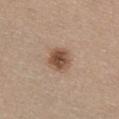Clinical impression:
No biopsy was performed on this lesion — it was imaged during a full skin examination and was not determined to be concerning.
Clinical summary:
A female patient aged around 65. Approximately 3.5 mm at its widest. A roughly 15 mm field-of-view crop from a total-body skin photograph. The lesion is located on the chest. Captured under white-light illumination. Automated tile analysis of the lesion measured an area of roughly 7.5 mm², an eccentricity of roughly 0.6, and two-axis asymmetry of about 0.2. It also reported a lesion-to-skin contrast of about 9.5 (normalized; higher = more distinct).